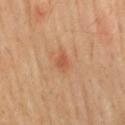{"biopsy_status": "not biopsied; imaged during a skin examination"}